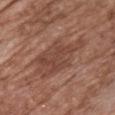Impression:
Imaged during a routine full-body skin examination; the lesion was not biopsied and no histopathology is available.
Acquisition and patient details:
Automated tile analysis of the lesion measured a mean CIELAB color near L≈44 a*≈21 b*≈27, roughly 7 lightness units darker than nearby skin, and a normalized lesion–skin contrast near 6. The analysis additionally found a nevus-likeness score of about 5/100. Measured at roughly 8 mm in maximum diameter. A female patient aged approximately 75. Located on the chest. Imaged with white-light lighting. A close-up tile cropped from a whole-body skin photograph, about 15 mm across.